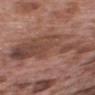* follow-up · imaged on a skin check; not biopsied
* imaging modality · total-body-photography crop, ~15 mm field of view
* subject · male, about 70 years old
* location · the upper back
* size · ≈10.5 mm
* TBP lesion metrics · an area of roughly 33 mm²; an average lesion color of about L≈45 a*≈20 b*≈25 (CIELAB), about 9 CIELAB-L* units darker than the surrounding skin, and a normalized border contrast of about 7; a border-irregularity rating of about 9.5/10, a within-lesion color-variation index near 5/10, and peripheral color asymmetry of about 1.5; a nevus-likeness score of about 0/100
* illumination · white-light